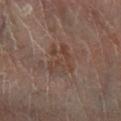biopsy status: imaged on a skin check; not biopsied
lighting: cross-polarized
TBP lesion metrics: a lesion area of about 10 mm²; lesion-presence confidence of about 100/100
diameter: ~4 mm (longest diameter)
patient: male, in their 70s
image source: ~15 mm crop, total-body skin-cancer survey
site: the right lower leg